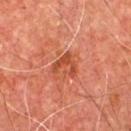A close-up tile cropped from a whole-body skin photograph, about 15 mm across. A male patient, in their mid- to late 60s. The lesion is on the chest. Longest diameter approximately 3 mm.